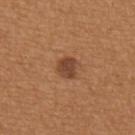Captured during whole-body skin photography for melanoma surveillance; the lesion was not biopsied.
Approximately 2.5 mm at its widest.
A female subject approximately 35 years of age.
Automated tile analysis of the lesion measured a lesion color around L≈44 a*≈22 b*≈32 in CIELAB, roughly 11 lightness units darker than nearby skin, and a normalized border contrast of about 8.5. And it measured a border-irregularity rating of about 1.5/10, internal color variation of about 3.5 on a 0–10 scale, and radial color variation of about 1.5. It also reported an automated nevus-likeness rating near 60 out of 100 and a lesion-detection confidence of about 100/100.
A 15 mm close-up tile from a total-body photography series done for melanoma screening.
The lesion is located on the upper back.